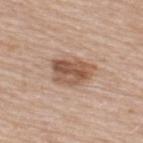Recorded during total-body skin imaging; not selected for excision or biopsy.
A close-up tile cropped from a whole-body skin photograph, about 15 mm across.
From the back.
A male subject, aged around 65.